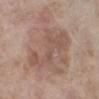Q: Was a biopsy performed?
A: imaged on a skin check; not biopsied
Q: Lesion location?
A: the leg
Q: How was the tile lit?
A: white-light illumination
Q: What is the imaging modality?
A: total-body-photography crop, ~15 mm field of view
Q: Patient demographics?
A: female, aged approximately 75
Q: How large is the lesion?
A: ≈7.5 mm